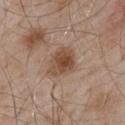Findings:
- biopsy status: total-body-photography surveillance lesion; no biopsy
- lighting: white-light illumination
- diameter: ~4 mm (longest diameter)
- subject: male, approximately 55 years of age
- image: ~15 mm crop, total-body skin-cancer survey
- TBP lesion metrics: a mean CIELAB color near L≈48 a*≈18 b*≈29 and a lesion–skin lightness drop of about 11; a detector confidence of about 100 out of 100 that the crop contains a lesion
- anatomic site: the mid back